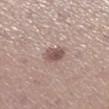{
  "biopsy_status": "not biopsied; imaged during a skin examination",
  "lesion_size": {
    "long_diameter_mm_approx": 2.5
  },
  "image": {
    "source": "total-body photography crop",
    "field_of_view_mm": 15
  },
  "patient": {
    "sex": "female",
    "age_approx": 35
  },
  "site": "right lower leg",
  "lighting": "white-light"
}